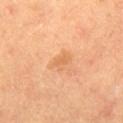Recorded during total-body skin imaging; not selected for excision or biopsy. A male subject, aged around 60. Captured under cross-polarized illumination. Cropped from a whole-body photographic skin survey; the tile spans about 15 mm. The lesion's longest dimension is about 3 mm. The total-body-photography lesion software estimated roughly 7 lightness units darker than nearby skin and a lesion-to-skin contrast of about 5 (normalized; higher = more distinct). The software also gave a border-irregularity index near 3/10 and peripheral color asymmetry of about 0.5. It also reported an automated nevus-likeness rating near 10 out of 100 and a lesion-detection confidence of about 100/100. The lesion is on the right thigh.